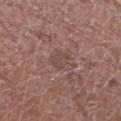biopsy status: no biopsy performed (imaged during a skin exam) | patient: male, in their mid- to late 70s | tile lighting: white-light illumination | image: ~15 mm crop, total-body skin-cancer survey | diameter: about 3 mm | image-analysis metrics: a footprint of about 4 mm², an eccentricity of roughly 0.8, and two-axis asymmetry of about 0.3; an average lesion color of about L≈46 a*≈18 b*≈21 (CIELAB), about 6 CIELAB-L* units darker than the surrounding skin, and a lesion-to-skin contrast of about 5 (normalized; higher = more distinct); border irregularity of about 3 on a 0–10 scale, a within-lesion color-variation index near 1.5/10, and radial color variation of about 0.5; a nevus-likeness score of about 0/100 | site: the left lower leg.